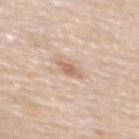biopsy status: total-body-photography surveillance lesion; no biopsy
site: the upper back
subject: female, aged approximately 50
image: 15 mm crop, total-body photography